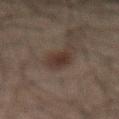diameter = ~3.5 mm (longest diameter) | illumination = cross-polarized illumination | patient = male, about 60 years old | body site = the abdomen | image source = 15 mm crop, total-body photography.